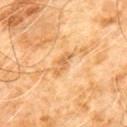An algorithmic analysis of the crop reported a lesion area of about 3.5 mm² and a shape eccentricity near 0.9. The analysis additionally found a border-irregularity index near 5/10 and a peripheral color-asymmetry measure near 0.5.
This is a cross-polarized tile.
The lesion's longest dimension is about 3 mm.
The lesion is on the chest.
A male subject aged around 60.
A lesion tile, about 15 mm wide, cut from a 3D total-body photograph.